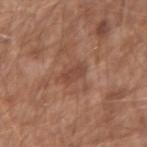Findings:
- workup: total-body-photography surveillance lesion; no biopsy
- tile lighting: white-light
- subject: male, aged approximately 65
- location: the left upper arm
- lesion size: ≈3 mm
- acquisition: ~15 mm crop, total-body skin-cancer survey